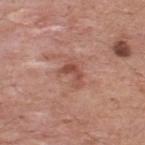The lesion was photographed on a routine skin check and not biopsied; there is no pathology result.
The lesion is on the upper back.
The patient is a male about 55 years old.
A lesion tile, about 15 mm wide, cut from a 3D total-body photograph.